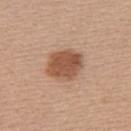| key | value |
|---|---|
| follow-up | imaged on a skin check; not biopsied |
| body site | the upper back |
| subject | female, roughly 40 years of age |
| diameter | ~4.5 mm (longest diameter) |
| image source | 15 mm crop, total-body photography |
| tile lighting | white-light |